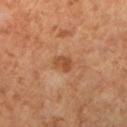  biopsy_status: not biopsied; imaged during a skin examination
  site: leg
  lesion_size:
    long_diameter_mm_approx: 2.5
  image:
    source: total-body photography crop
    field_of_view_mm: 15
  lighting: cross-polarized
  patient:
    sex: female
    age_approx: 65
  automated_metrics:
    eccentricity: 0.75
    cielab_L: 48
    cielab_a: 24
    cielab_b: 36
    vs_skin_contrast_norm: 7.0
    color_variation_0_10: 3.5
    peripheral_color_asymmetry: 1.5
    nevus_likeness_0_100: 5
    lesion_detection_confidence_0_100: 100